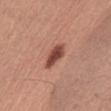<record>
<biopsy_status>not biopsied; imaged during a skin examination</biopsy_status>
<patient>
  <sex>male</sex>
  <age_approx>45</age_approx>
</patient>
<lesion_size>
  <long_diameter_mm_approx>3.5</long_diameter_mm_approx>
</lesion_size>
<lighting>white-light</lighting>
<site>right upper arm</site>
<image>
  <source>total-body photography crop</source>
  <field_of_view_mm>15</field_of_view_mm>
</image>
</record>